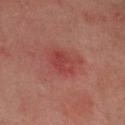Context:
The lesion is located on the head or neck. Automated image analysis of the tile measured a lesion area of about 6 mm², an eccentricity of roughly 0.35, and a symmetry-axis asymmetry near 0.2. The analysis additionally found a lesion color around L≈37 a*≈29 b*≈23 in CIELAB, roughly 6 lightness units darker than nearby skin, and a normalized border contrast of about 5.5. The software also gave a within-lesion color-variation index near 3/10 and peripheral color asymmetry of about 1. A close-up tile cropped from a whole-body skin photograph, about 15 mm across. This is a cross-polarized tile. The patient is a male aged 58 to 62.